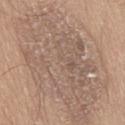The lesion was tiled from a total-body skin photograph and was not biopsied. The subject is a male roughly 60 years of age. A 15 mm close-up extracted from a 3D total-body photography capture. From the leg.